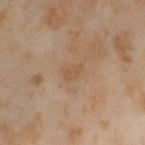biopsy_status: not biopsied; imaged during a skin examination
site: left thigh
automated_metrics:
  area_mm2_approx: 3.0
  cielab_L: 54
  cielab_a: 17
  cielab_b: 33
  vs_skin_darker_L: 6.0
  vs_skin_contrast_norm: 5.0
  border_irregularity_0_10: 3.5
  nevus_likeness_0_100: 0
  lesion_detection_confidence_0_100: 100
lesion_size:
  long_diameter_mm_approx: 2.5
image:
  source: total-body photography crop
  field_of_view_mm: 15
lighting: cross-polarized
patient:
  sex: female
  age_approx: 55